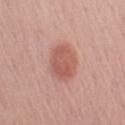<lesion>
  <biopsy_status>not biopsied; imaged during a skin examination</biopsy_status>
  <patient>
    <sex>male</sex>
    <age_approx>60</age_approx>
  </patient>
  <image>
    <source>total-body photography crop</source>
    <field_of_view_mm>15</field_of_view_mm>
  </image>
  <site>left upper arm</site>
</lesion>